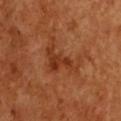Case summary:
* workup — no biopsy performed (imaged during a skin exam)
* subject — female, in their mid- to late 50s
* image source — 15 mm crop, total-body photography
* body site — the chest
* automated lesion analysis — a lesion area of about 12 mm², an outline eccentricity of about 0.85 (0 = round, 1 = elongated), and two-axis asymmetry of about 0.65; an average lesion color of about L≈39 a*≈28 b*≈37 (CIELAB), about 8 CIELAB-L* units darker than the surrounding skin, and a normalized border contrast of about 6.5
* diameter — about 6 mm
* illumination — cross-polarized